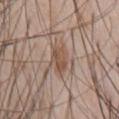{"biopsy_status": "not biopsied; imaged during a skin examination", "image": {"source": "total-body photography crop", "field_of_view_mm": 15}, "lesion_size": {"long_diameter_mm_approx": 3.0}, "automated_metrics": {"eccentricity": 0.7, "shape_asymmetry": 0.25, "nevus_likeness_0_100": 75, "lesion_detection_confidence_0_100": 95}, "site": "chest", "patient": {"sex": "male", "age_approx": 60}}